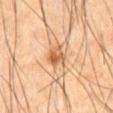The lesion is located on the abdomen.
A male subject aged around 65.
Cropped from a whole-body photographic skin survey; the tile spans about 15 mm.